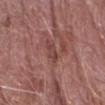Captured during whole-body skin photography for melanoma surveillance; the lesion was not biopsied.
A male patient, in their 80s.
The lesion is located on the right forearm.
A 15 mm close-up extracted from a 3D total-body photography capture.